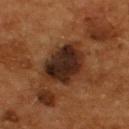Findings:
- notes · catalogued during a skin exam; not biopsied
- image · total-body-photography crop, ~15 mm field of view
- subject · male, aged approximately 55
- lesion diameter · about 6.5 mm
- image-analysis metrics · an area of roughly 20 mm² and an outline eccentricity of about 0.75 (0 = round, 1 = elongated); about 13 CIELAB-L* units darker than the surrounding skin
- illumination · cross-polarized illumination
- anatomic site · the upper back